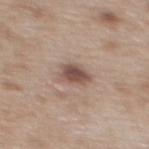Context:
From the upper back. An algorithmic analysis of the crop reported a lesion area of about 5.5 mm², an outline eccentricity of about 0.6 (0 = round, 1 = elongated), and a shape-asymmetry score of about 0.2 (0 = symmetric). It also reported internal color variation of about 3 on a 0–10 scale and radial color variation of about 1. Cropped from a whole-body photographic skin survey; the tile spans about 15 mm. A female subject, approximately 40 years of age.